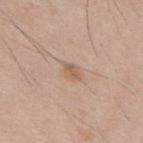Clinical impression: Imaged during a routine full-body skin examination; the lesion was not biopsied and no histopathology is available. Acquisition and patient details: A male patient, approximately 35 years of age. The lesion is located on the back. Cropped from a total-body skin-imaging series; the visible field is about 15 mm.